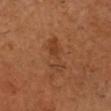| field | value |
|---|---|
| follow-up | catalogued during a skin exam; not biopsied |
| lighting | cross-polarized illumination |
| imaging modality | total-body-photography crop, ~15 mm field of view |
| location | the head or neck |
| subject | male, aged approximately 50 |
| size | ≈5.5 mm |
| image-analysis metrics | a mean CIELAB color near L≈40 a*≈23 b*≈34, a lesion–skin lightness drop of about 7, and a normalized lesion–skin contrast near 5.5 |